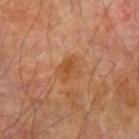<case>
  <biopsy_status>not biopsied; imaged during a skin examination</biopsy_status>
  <image>
    <source>total-body photography crop</source>
    <field_of_view_mm>15</field_of_view_mm>
  </image>
  <patient>
    <sex>male</sex>
    <age_approx>70</age_approx>
  </patient>
  <lighting>cross-polarized</lighting>
  <lesion_size>
    <long_diameter_mm_approx>3.0</long_diameter_mm_approx>
  </lesion_size>
  <site>left forearm</site>
</case>